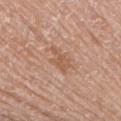Part of a total-body skin-imaging series; this lesion was reviewed on a skin check and was not flagged for biopsy.